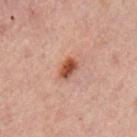anatomic site: the left upper arm
TBP lesion metrics: a lesion color around L≈41 a*≈22 b*≈27 in CIELAB, about 12 CIELAB-L* units darker than the surrounding skin, and a lesion-to-skin contrast of about 10.5 (normalized; higher = more distinct); a border-irregularity rating of about 1.5/10 and peripheral color asymmetry of about 1; a detector confidence of about 100 out of 100 that the crop contains a lesion
image: total-body-photography crop, ~15 mm field of view
subject: male, aged approximately 30
lesion diameter: ~2.5 mm (longest diameter)
illumination: cross-polarized